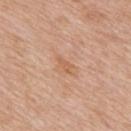biopsy status: catalogued during a skin exam; not biopsied | subject: male, about 60 years old | imaging modality: ~15 mm crop, total-body skin-cancer survey | tile lighting: white-light | automated metrics: border irregularity of about 3 on a 0–10 scale, a color-variation rating of about 0/10, and radial color variation of about 0 | lesion size: ≈2.5 mm | body site: the mid back.